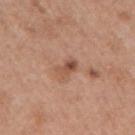Imaged during a routine full-body skin examination; the lesion was not biopsied and no histopathology is available.
Captured under white-light illumination.
A close-up tile cropped from a whole-body skin photograph, about 15 mm across.
A female subject, approximately 40 years of age.
The lesion-visualizer software estimated a mean CIELAB color near L≈51 a*≈22 b*≈29, a lesion–skin lightness drop of about 10, and a normalized lesion–skin contrast near 7. It also reported border irregularity of about 3 on a 0–10 scale, internal color variation of about 8 on a 0–10 scale, and peripheral color asymmetry of about 3.
On the right upper arm.